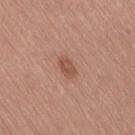illumination: white-light illumination | diameter: about 3 mm | automated lesion analysis: an outline eccentricity of about 0.8 (0 = round, 1 = elongated); roughly 9 lightness units darker than nearby skin and a normalized lesion–skin contrast near 6.5 | subject: female, aged approximately 60 | site: the leg | acquisition: total-body-photography crop, ~15 mm field of view.